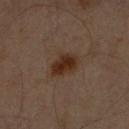• subject: male, in their 60s
• lesion size: ≈4 mm
• image: ~15 mm crop, total-body skin-cancer survey
• location: the right forearm